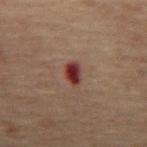Background: A male subject, aged 68 to 72. Measured at roughly 3 mm in maximum diameter. The lesion is located on the mid back. This image is a 15 mm lesion crop taken from a total-body photograph.